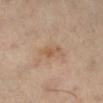Part of a total-body skin-imaging series; this lesion was reviewed on a skin check and was not flagged for biopsy. A female patient in their 50s. The tile uses cross-polarized illumination. The lesion's longest dimension is about 4.5 mm. From the right lower leg. The lesion-visualizer software estimated an average lesion color of about L≈55 a*≈16 b*≈31 (CIELAB) and a normalized lesion–skin contrast near 5.5. A 15 mm close-up tile from a total-body photography series done for melanoma screening.